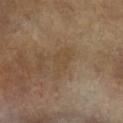Clinical impression: The lesion was photographed on a routine skin check and not biopsied; there is no pathology result. Background: Captured under cross-polarized illumination. A 15 mm close-up extracted from a 3D total-body photography capture. The lesion's longest dimension is about 4.5 mm. On the arm. The subject is a female approximately 75 years of age. The total-body-photography lesion software estimated a classifier nevus-likeness of about 0/100 and a lesion-detection confidence of about 100/100.